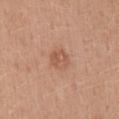Findings:
- workup — total-body-photography surveillance lesion; no biopsy
- image source — ~15 mm crop, total-body skin-cancer survey
- tile lighting — white-light illumination
- subject — male, approximately 30 years of age
- body site — the mid back
- size — ~2.5 mm (longest diameter)